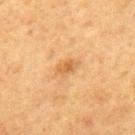Q: Is there a histopathology result?
A: imaged on a skin check; not biopsied
Q: Patient demographics?
A: male, aged 73 to 77
Q: Where on the body is the lesion?
A: the mid back
Q: How was this image acquired?
A: total-body-photography crop, ~15 mm field of view
Q: Illumination type?
A: cross-polarized